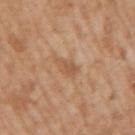body site = the right upper arm
imaging modality = 15 mm crop, total-body photography
subject = male, in their mid-60s